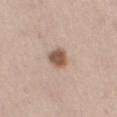Imaged during a routine full-body skin examination; the lesion was not biopsied and no histopathology is available. Captured under white-light illumination. A male subject aged 63–67. The lesion is on the left thigh. Longest diameter approximately 2.5 mm. This image is a 15 mm lesion crop taken from a total-body photograph. The lesion-visualizer software estimated a mean CIELAB color near L≈54 a*≈18 b*≈26, a lesion–skin lightness drop of about 15, and a normalized lesion–skin contrast near 10. The software also gave a nevus-likeness score of about 95/100 and lesion-presence confidence of about 100/100.